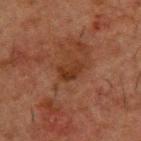| key | value |
|---|---|
| biopsy status | total-body-photography surveillance lesion; no biopsy |
| patient | male, roughly 50 years of age |
| anatomic site | the upper back |
| imaging modality | ~15 mm tile from a whole-body skin photo |
| lesion diameter | ≈3 mm |